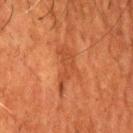Q: Is there a histopathology result?
A: catalogued during a skin exam; not biopsied
Q: Lesion location?
A: the head or neck
Q: Lesion size?
A: ~5.5 mm (longest diameter)
Q: What is the imaging modality?
A: 15 mm crop, total-body photography
Q: Patient demographics?
A: male, in their 50s
Q: What lighting was used for the tile?
A: cross-polarized illumination